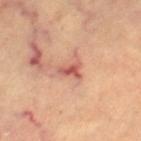The lesion was tiled from a total-body skin photograph and was not biopsied. Located on the leg. A region of skin cropped from a whole-body photographic capture, roughly 15 mm wide. The subject is aged 58–62. Longest diameter approximately 3 mm. An algorithmic analysis of the crop reported a lesion–skin lightness drop of about 12 and a normalized border contrast of about 7.5. The analysis additionally found a border-irregularity rating of about 4.5/10, internal color variation of about 3.5 on a 0–10 scale, and radial color variation of about 1. And it measured a nevus-likeness score of about 0/100 and lesion-presence confidence of about 100/100.